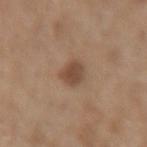Part of a total-body skin-imaging series; this lesion was reviewed on a skin check and was not flagged for biopsy. Imaged with white-light lighting. The lesion is located on the right forearm. A roughly 15 mm field-of-view crop from a total-body skin photograph. A male patient roughly 70 years of age. About 3 mm across.